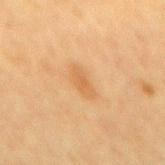Recorded during total-body skin imaging; not selected for excision or biopsy. The lesion is on the mid back. About 4 mm across. Captured under cross-polarized illumination. The subject is a female aged around 55. A 15 mm close-up tile from a total-body photography series done for melanoma screening.